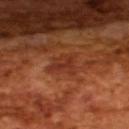The lesion was photographed on a routine skin check and not biopsied; there is no pathology result. About 3 mm across. A male patient, aged approximately 65. A 15 mm close-up extracted from a 3D total-body photography capture. Automated tile analysis of the lesion measured a footprint of about 6.5 mm² and an eccentricity of roughly 0.3. The analysis additionally found a border-irregularity rating of about 4.5/10, a color-variation rating of about 3/10, and peripheral color asymmetry of about 1. The analysis additionally found an automated nevus-likeness rating near 0 out of 100. This is a cross-polarized tile.